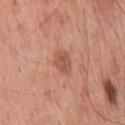Notes:
– lighting: white-light
– image: ~15 mm crop, total-body skin-cancer survey
– site: the back
– patient: male, aged 53 to 57
– automated lesion analysis: a lesion area of about 4.5 mm² and an eccentricity of roughly 0.7; an average lesion color of about L≈54 a*≈26 b*≈32 (CIELAB) and a lesion–skin lightness drop of about 9; a nevus-likeness score of about 65/100
– lesion diameter: ≈3 mm